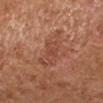biopsy_status: not biopsied; imaged during a skin examination
patient:
  sex: female
  age_approx: 50
image:
  source: total-body photography crop
  field_of_view_mm: 15
site: arm